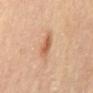Imaged during a routine full-body skin examination; the lesion was not biopsied and no histopathology is available.
Longest diameter approximately 3.5 mm.
A male patient aged around 85 or older.
The lesion-visualizer software estimated a lesion color around L≈58 a*≈22 b*≈34 in CIELAB and about 10 CIELAB-L* units darker than the surrounding skin. And it measured a classifier nevus-likeness of about 90/100 and lesion-presence confidence of about 100/100.
This is a cross-polarized tile.
A close-up tile cropped from a whole-body skin photograph, about 15 mm across.
On the mid back.